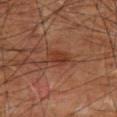The recorded lesion diameter is about 3.5 mm.
A male subject aged 58–62.
The lesion is located on the mid back.
A region of skin cropped from a whole-body photographic capture, roughly 15 mm wide.
The tile uses cross-polarized illumination.